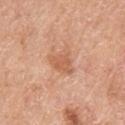Findings:
• notes: total-body-photography surveillance lesion; no biopsy
• automated lesion analysis: a lesion color around L≈60 a*≈25 b*≈35 in CIELAB, about 9 CIELAB-L* units darker than the surrounding skin, and a normalized border contrast of about 6; a border-irregularity rating of about 4/10, internal color variation of about 1.5 on a 0–10 scale, and radial color variation of about 0.5
• size: ≈3 mm
• body site: the back
• subject: male, aged approximately 60
• tile lighting: white-light illumination
• image source: ~15 mm crop, total-body skin-cancer survey